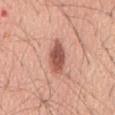Part of a total-body skin-imaging series; this lesion was reviewed on a skin check and was not flagged for biopsy. The lesion-visualizer software estimated a footprint of about 8 mm², an outline eccentricity of about 0.9 (0 = round, 1 = elongated), and two-axis asymmetry of about 0.2. The analysis additionally found an automated nevus-likeness rating near 90 out of 100. From the abdomen. A region of skin cropped from a whole-body photographic capture, roughly 15 mm wide. Imaged with white-light lighting. Measured at roughly 5 mm in maximum diameter. A male subject, roughly 60 years of age.